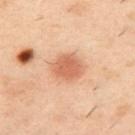| key | value |
|---|---|
| follow-up | imaged on a skin check; not biopsied |
| tile lighting | cross-polarized |
| image source | ~15 mm tile from a whole-body skin photo |
| subject | male, in their 40s |
| size | about 4 mm |
| location | the back |